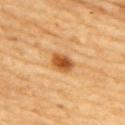Imaged during a routine full-body skin examination; the lesion was not biopsied and no histopathology is available.
On the upper back.
The subject is a female aged approximately 70.
This image is a 15 mm lesion crop taken from a total-body photograph.
Measured at roughly 3 mm in maximum diameter.
Imaged with cross-polarized lighting.
The total-body-photography lesion software estimated a footprint of about 5 mm² and two-axis asymmetry of about 0.2. It also reported a classifier nevus-likeness of about 100/100.